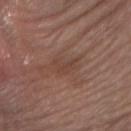Findings:
– follow-up: total-body-photography surveillance lesion; no biopsy
– subject: male, aged approximately 65
– image: ~15 mm crop, total-body skin-cancer survey
– body site: the arm